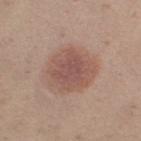workup: total-body-photography surveillance lesion; no biopsy | image source: ~15 mm tile from a whole-body skin photo | location: the leg | patient: female, aged approximately 30 | automated metrics: a border-irregularity rating of about 1.5/10, internal color variation of about 3.5 on a 0–10 scale, and peripheral color asymmetry of about 1.